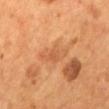Case summary:
– notes · imaged on a skin check; not biopsied
– patient · male, aged approximately 55
– acquisition · ~15 mm tile from a whole-body skin photo
– body site · the mid back